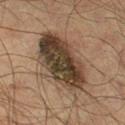Context:
Automated tile analysis of the lesion measured a footprint of about 34 mm², a shape eccentricity near 0.8, and a symmetry-axis asymmetry near 0.2. And it measured a normalized border contrast of about 11.5. And it measured a color-variation rating of about 9/10 and a peripheral color-asymmetry measure near 3. The analysis additionally found a detector confidence of about 95 out of 100 that the crop contains a lesion. The subject is a male roughly 55 years of age. From the right lower leg. Measured at roughly 9 mm in maximum diameter. This is a cross-polarized tile. A 15 mm close-up extracted from a 3D total-body photography capture.